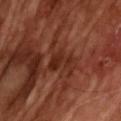Imaged during a routine full-body skin examination; the lesion was not biopsied and no histopathology is available. A 15 mm crop from a total-body photograph taken for skin-cancer surveillance. The subject is a male aged around 65. Automated tile analysis of the lesion measured an area of roughly 5.5 mm², an outline eccentricity of about 0.6 (0 = round, 1 = elongated), and a shape-asymmetry score of about 0.4 (0 = symmetric). It also reported a mean CIELAB color near L≈28 a*≈25 b*≈28, a lesion–skin lightness drop of about 7, and a lesion-to-skin contrast of about 6.5 (normalized; higher = more distinct). The analysis additionally found internal color variation of about 1 on a 0–10 scale. And it measured a lesion-detection confidence of about 90/100. Imaged with cross-polarized lighting.